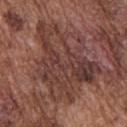<case>
  <biopsy_status>not biopsied; imaged during a skin examination</biopsy_status>
  <image>
    <source>total-body photography crop</source>
    <field_of_view_mm>15</field_of_view_mm>
  </image>
  <patient>
    <sex>male</sex>
    <age_approx>75</age_approx>
  </patient>
  <lighting>white-light</lighting>
  <lesion_size>
    <long_diameter_mm_approx>9.5</long_diameter_mm_approx>
  </lesion_size>
  <site>upper back</site>
</case>